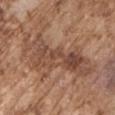Q: Is there a histopathology result?
A: catalogued during a skin exam; not biopsied
Q: How large is the lesion?
A: ≈10 mm
Q: What is the imaging modality?
A: 15 mm crop, total-body photography
Q: Illumination type?
A: white-light
Q: Who is the patient?
A: male, approximately 75 years of age
Q: Automated lesion metrics?
A: about 10 CIELAB-L* units darker than the surrounding skin and a normalized border contrast of about 7; a border-irregularity index near 6/10, a within-lesion color-variation index near 6.5/10, and peripheral color asymmetry of about 2.5
Q: Lesion location?
A: the right upper arm